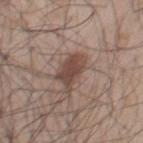{
  "biopsy_status": "not biopsied; imaged during a skin examination",
  "lesion_size": {
    "long_diameter_mm_approx": 4.5
  },
  "patient": {
    "sex": "male",
    "age_approx": 55
  },
  "site": "chest",
  "image": {
    "source": "total-body photography crop",
    "field_of_view_mm": 15
  },
  "automated_metrics": {
    "eccentricity": 0.85,
    "vs_skin_darker_L": 11.0,
    "vs_skin_contrast_norm": 9.0,
    "nevus_likeness_0_100": 95,
    "lesion_detection_confidence_0_100": 100
  },
  "lighting": "white-light"
}